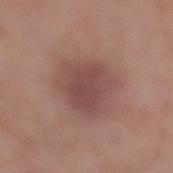Imaged during a routine full-body skin examination; the lesion was not biopsied and no histopathology is available. The subject is a female roughly 55 years of age. Measured at roughly 6.5 mm in maximum diameter. Cropped from a total-body skin-imaging series; the visible field is about 15 mm. The lesion is located on the leg. The lesion-visualizer software estimated an area of roughly 21 mm², an eccentricity of roughly 0.7, and a symmetry-axis asymmetry near 0.25. The software also gave an average lesion color of about L≈47 a*≈21 b*≈22 (CIELAB), a lesion–skin lightness drop of about 9, and a normalized border contrast of about 7. The analysis additionally found a within-lesion color-variation index near 3/10.